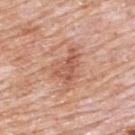workup: catalogued during a skin exam; not biopsied | image: ~15 mm tile from a whole-body skin photo | subject: male, aged 78 to 82 | anatomic site: the back | automated metrics: a lesion area of about 8 mm², an outline eccentricity of about 0.8 (0 = round, 1 = elongated), and two-axis asymmetry of about 0.5; an average lesion color of about L≈57 a*≈25 b*≈31 (CIELAB) and a lesion–skin lightness drop of about 10; an automated nevus-likeness rating near 10 out of 100 and a lesion-detection confidence of about 100/100 | tile lighting: white-light illumination | diameter: ≈4 mm.